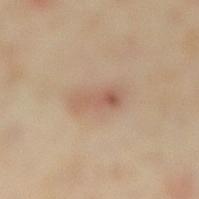Q: Was this lesion biopsied?
A: total-body-photography surveillance lesion; no biopsy
Q: Illumination type?
A: cross-polarized
Q: How large is the lesion?
A: ~4 mm (longest diameter)
Q: What is the anatomic site?
A: the right lower leg
Q: What are the patient's age and sex?
A: female, in their mid-30s
Q: What is the imaging modality?
A: total-body-photography crop, ~15 mm field of view